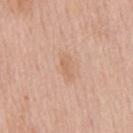Assessment: Part of a total-body skin-imaging series; this lesion was reviewed on a skin check and was not flagged for biopsy. Image and clinical context: Captured under white-light illumination. The lesion-visualizer software estimated a lesion area of about 2.5 mm², an outline eccentricity of about 0.9 (0 = round, 1 = elongated), and a shape-asymmetry score of about 0.45 (0 = symmetric). The software also gave a lesion color around L≈64 a*≈20 b*≈32 in CIELAB, a lesion–skin lightness drop of about 6, and a lesion-to-skin contrast of about 5 (normalized; higher = more distinct). It also reported a border-irregularity index near 4.5/10, a color-variation rating of about 0/10, and radial color variation of about 0. From the chest. Approximately 3 mm at its widest. Cropped from a total-body skin-imaging series; the visible field is about 15 mm. The subject is a male aged 58–62.